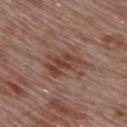This lesion was catalogued during total-body skin photography and was not selected for biopsy. About 5 mm across. The lesion is located on the upper back. The patient is a male aged 53 to 57. A close-up tile cropped from a whole-body skin photograph, about 15 mm across.